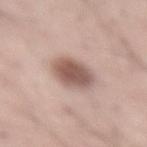biopsy_status: not biopsied; imaged during a skin examination
lesion_size:
  long_diameter_mm_approx: 4.5
lighting: white-light
site: lower back
image:
  source: total-body photography crop
  field_of_view_mm: 15
patient:
  sex: male
  age_approx: 55